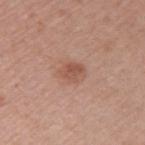Part of a total-body skin-imaging series; this lesion was reviewed on a skin check and was not flagged for biopsy. Automated tile analysis of the lesion measured a footprint of about 5 mm², an eccentricity of roughly 0.65, and two-axis asymmetry of about 0.15. And it measured an average lesion color of about L≈53 a*≈22 b*≈28 (CIELAB), roughly 9 lightness units darker than nearby skin, and a lesion-to-skin contrast of about 6.5 (normalized; higher = more distinct). Cropped from a total-body skin-imaging series; the visible field is about 15 mm. The lesion is on the left upper arm. A female patient, roughly 45 years of age.